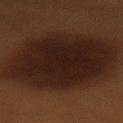Impression: Imaged during a routine full-body skin examination; the lesion was not biopsied and no histopathology is available. Context: A 15 mm close-up extracted from a 3D total-body photography capture. The tile uses cross-polarized illumination. About 11.5 mm across. Automated tile analysis of the lesion measured a lesion color around L≈18 a*≈15 b*≈20 in CIELAB, a lesion–skin lightness drop of about 9, and a lesion-to-skin contrast of about 12 (normalized; higher = more distinct). The analysis additionally found border irregularity of about 2 on a 0–10 scale and a within-lesion color-variation index near 3.5/10. The analysis additionally found a classifier nevus-likeness of about 45/100 and lesion-presence confidence of about 100/100. The lesion is located on the lower back. A female patient, aged approximately 30.